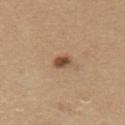Part of a total-body skin-imaging series; this lesion was reviewed on a skin check and was not flagged for biopsy.
The subject is a female about 60 years old.
From the upper back.
The lesion-visualizer software estimated an average lesion color of about L≈49 a*≈18 b*≈32 (CIELAB) and a lesion-to-skin contrast of about 10 (normalized; higher = more distinct). And it measured a within-lesion color-variation index near 2/10 and radial color variation of about 0.5. The software also gave a classifier nevus-likeness of about 95/100.
The lesion's longest dimension is about 2.5 mm.
Cropped from a total-body skin-imaging series; the visible field is about 15 mm.